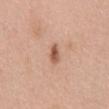<tbp_lesion>
  <site>abdomen</site>
  <lesion_size>
    <long_diameter_mm_approx>3.0</long_diameter_mm_approx>
  </lesion_size>
  <image>
    <source>total-body photography crop</source>
    <field_of_view_mm>15</field_of_view_mm>
  </image>
  <patient>
    <sex>male</sex>
    <age_approx>60</age_approx>
  </patient>
</tbp_lesion>